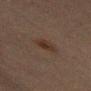Notes:
• biopsy status — imaged on a skin check; not biopsied
• lesion size — about 3 mm
• TBP lesion metrics — a border-irregularity index near 2.5/10 and a within-lesion color-variation index near 2/10; a classifier nevus-likeness of about 80/100 and a detector confidence of about 100 out of 100 that the crop contains a lesion
• patient — male, aged around 70
• anatomic site — the back
• imaging modality — ~15 mm tile from a whole-body skin photo